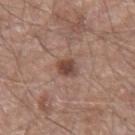| field | value |
|---|---|
| follow-up | no biopsy performed (imaged during a skin exam) |
| image | 15 mm crop, total-body photography |
| lighting | white-light |
| patient | male, aged around 60 |
| site | the arm |